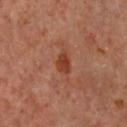{
  "biopsy_status": "not biopsied; imaged during a skin examination",
  "lesion_size": {
    "long_diameter_mm_approx": 2.5
  },
  "image": {
    "source": "total-body photography crop",
    "field_of_view_mm": 15
  },
  "patient": {
    "sex": "female",
    "age_approx": 60
  },
  "lighting": "cross-polarized",
  "site": "chest"
}